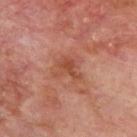* subject: male, aged approximately 70
* body site: the upper back
* imaging modality: total-body-photography crop, ~15 mm field of view